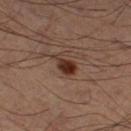Captured during whole-body skin photography for melanoma surveillance; the lesion was not biopsied. Located on the left thigh. Approximately 3.5 mm at its widest. Captured under cross-polarized illumination. The subject is a male in their 60s. Cropped from a whole-body photographic skin survey; the tile spans about 15 mm. Automated tile analysis of the lesion measured a lesion area of about 4.5 mm², an outline eccentricity of about 0.8 (0 = round, 1 = elongated), and two-axis asymmetry of about 0.4. The analysis additionally found border irregularity of about 4.5 on a 0–10 scale, internal color variation of about 3.5 on a 0–10 scale, and a peripheral color-asymmetry measure near 1.